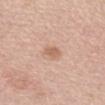Impression: Part of a total-body skin-imaging series; this lesion was reviewed on a skin check and was not flagged for biopsy. Clinical summary: Located on the mid back. Imaged with white-light lighting. A female subject aged 63 to 67. A 15 mm close-up tile from a total-body photography series done for melanoma screening.